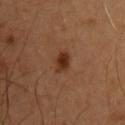Case summary:
– biopsy status · imaged on a skin check; not biopsied
– anatomic site · the chest
– patient · male, in their mid-50s
– acquisition · 15 mm crop, total-body photography